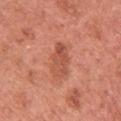Part of a total-body skin-imaging series; this lesion was reviewed on a skin check and was not flagged for biopsy.
On the left upper arm.
Longest diameter approximately 4 mm.
A 15 mm crop from a total-body photograph taken for skin-cancer surveillance.
The tile uses white-light illumination.
A female patient approximately 50 years of age.